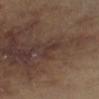The lesion was tiled from a total-body skin photograph and was not biopsied. The recorded lesion diameter is about 3 mm. The patient is aged approximately 60. A roughly 15 mm field-of-view crop from a total-body skin photograph. From the right lower leg. Captured under cross-polarized illumination. An algorithmic analysis of the crop reported a border-irregularity rating of about 5/10 and peripheral color asymmetry of about 0.